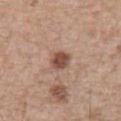Q: Was this lesion biopsied?
A: imaged on a skin check; not biopsied
Q: What is the lesion's diameter?
A: ~2.5 mm (longest diameter)
Q: Automated lesion metrics?
A: a footprint of about 5 mm², a shape eccentricity near 0.4, and a shape-asymmetry score of about 0.15 (0 = symmetric); an average lesion color of about L≈49 a*≈20 b*≈27 (CIELAB), a lesion–skin lightness drop of about 13, and a normalized lesion–skin contrast near 9.5
Q: What is the anatomic site?
A: the abdomen
Q: How was this image acquired?
A: ~15 mm tile from a whole-body skin photo
Q: Patient demographics?
A: male, approximately 70 years of age